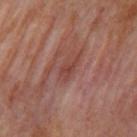Part of a total-body skin-imaging series; this lesion was reviewed on a skin check and was not flagged for biopsy. A region of skin cropped from a whole-body photographic capture, roughly 15 mm wide. Automated tile analysis of the lesion measured an area of roughly 3.5 mm² and an eccentricity of roughly 0.9. The software also gave an average lesion color of about L≈41 a*≈24 b*≈24 (CIELAB), about 7 CIELAB-L* units darker than the surrounding skin, and a normalized border contrast of about 6. The subject is a female aged 48 to 52. The lesion's longest dimension is about 3.5 mm. From the right upper arm.